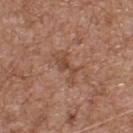Assessment: Recorded during total-body skin imaging; not selected for excision or biopsy. Context: The lesion is on the upper back. This image is a 15 mm lesion crop taken from a total-body photograph. Approximately 3.5 mm at its widest. The subject is a male aged around 55. Captured under white-light illumination.